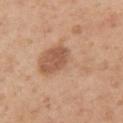Imaged during a routine full-body skin examination; the lesion was not biopsied and no histopathology is available. About 7 mm across. Imaged with white-light lighting. The lesion is located on the left upper arm. A 15 mm close-up tile from a total-body photography series done for melanoma screening. Automated image analysis of the tile measured a lesion area of about 21 mm² and a symmetry-axis asymmetry near 0.55. A female subject, approximately 40 years of age.